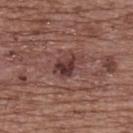subject — female, about 75 years old | lesion diameter — ≈3 mm | image source — ~15 mm crop, total-body skin-cancer survey | site — the upper back | TBP lesion metrics — a mean CIELAB color near L≈36 a*≈22 b*≈20, about 11 CIELAB-L* units darker than the surrounding skin, and a normalized lesion–skin contrast near 9.5; an automated nevus-likeness rating near 25 out of 100 and a lesion-detection confidence of about 100/100.